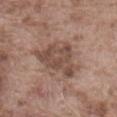This lesion was catalogued during total-body skin photography and was not selected for biopsy. The total-body-photography lesion software estimated a lesion area of about 16 mm², an outline eccentricity of about 0.7 (0 = round, 1 = elongated), and a shape-asymmetry score of about 0.4 (0 = symmetric). It also reported a lesion color around L≈48 a*≈18 b*≈24 in CIELAB, roughly 10 lightness units darker than nearby skin, and a lesion-to-skin contrast of about 7.5 (normalized; higher = more distinct). It also reported a nevus-likeness score of about 0/100 and a lesion-detection confidence of about 100/100. A 15 mm close-up extracted from a 3D total-body photography capture. The recorded lesion diameter is about 6 mm. On the abdomen. A male subject about 75 years old.